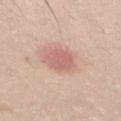follow-up: total-body-photography surveillance lesion; no biopsy
lesion diameter: ~3.5 mm (longest diameter)
location: the mid back
acquisition: ~15 mm crop, total-body skin-cancer survey
illumination: white-light illumination
subject: male, aged 23–27
automated metrics: internal color variation of about 2 on a 0–10 scale and radial color variation of about 0.5